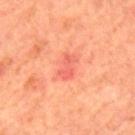A male patient, in their mid- to late 60s.
Cropped from a total-body skin-imaging series; the visible field is about 15 mm.
The tile uses cross-polarized illumination.
On the back.